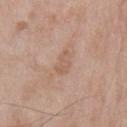Recorded during total-body skin imaging; not selected for excision or biopsy.
On the chest.
Automated image analysis of the tile measured a border-irregularity index near 3/10 and a within-lesion color-variation index near 2.5/10. And it measured a classifier nevus-likeness of about 0/100 and a lesion-detection confidence of about 100/100.
Cropped from a whole-body photographic skin survey; the tile spans about 15 mm.
Imaged with white-light lighting.
A male patient, approximately 65 years of age.